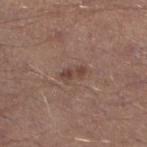The lesion was photographed on a routine skin check and not biopsied; there is no pathology result.
The subject is a male about 40 years old.
About 3 mm across.
On the left lower leg.
The tile uses white-light illumination.
A lesion tile, about 15 mm wide, cut from a 3D total-body photograph.
An algorithmic analysis of the crop reported a footprint of about 3.5 mm² and an eccentricity of roughly 0.9.